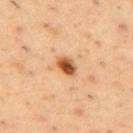The lesion is located on the upper back. A region of skin cropped from a whole-body photographic capture, roughly 15 mm wide. The patient is a male approximately 55 years of age. This is a cross-polarized tile. About 2.5 mm across.